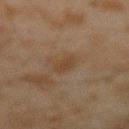From the right forearm.
A male patient about 45 years old.
A 15 mm close-up extracted from a 3D total-body photography capture.